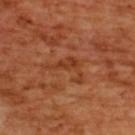Context:
Captured under cross-polarized illumination. On the upper back. Cropped from a whole-body photographic skin survey; the tile spans about 15 mm. A female patient, aged around 55.